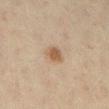Findings:
- workup · total-body-photography surveillance lesion; no biopsy
- image-analysis metrics · a lesion–skin lightness drop of about 9 and a normalized border contrast of about 7.5; a within-lesion color-variation index near 2/10 and radial color variation of about 0.5; a classifier nevus-likeness of about 95/100 and lesion-presence confidence of about 100/100
- patient · female, aged 43–47
- tile lighting · cross-polarized
- imaging modality · ~15 mm crop, total-body skin-cancer survey
- anatomic site · the left lower leg